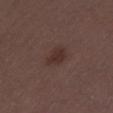Clinical impression: No biopsy was performed on this lesion — it was imaged during a full skin examination and was not determined to be concerning. Image and clinical context: The lesion is located on the left thigh. A female patient aged 28 to 32. Cropped from a whole-body photographic skin survey; the tile spans about 15 mm.